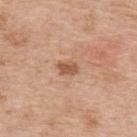Recorded during total-body skin imaging; not selected for excision or biopsy. Approximately 2.5 mm at its widest. The total-body-photography lesion software estimated an average lesion color of about L≈55 a*≈22 b*≈33 (CIELAB), roughly 11 lightness units darker than nearby skin, and a normalized border contrast of about 7.5. The software also gave border irregularity of about 2 on a 0–10 scale, a within-lesion color-variation index near 1.5/10, and peripheral color asymmetry of about 0.5. A roughly 15 mm field-of-view crop from a total-body skin photograph. A male patient about 70 years old. From the upper back.